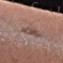The subject is a female roughly 25 years of age.
Captured under white-light illumination.
The total-body-photography lesion software estimated a lesion area of about 6.5 mm², a shape eccentricity near 0.95, and a shape-asymmetry score of about 0.25 (0 = symmetric). And it measured a mean CIELAB color near L≈48 a*≈16 b*≈21 and a normalized border contrast of about 6. It also reported a classifier nevus-likeness of about 0/100 and a detector confidence of about 90 out of 100 that the crop contains a lesion.
A lesion tile, about 15 mm wide, cut from a 3D total-body photograph.
From the right forearm.